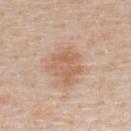Assessment: Captured during whole-body skin photography for melanoma surveillance; the lesion was not biopsied. Context: The tile uses white-light illumination. The patient is a male roughly 60 years of age. A 15 mm close-up tile from a total-body photography series done for melanoma screening. Measured at roughly 4.5 mm in maximum diameter. The lesion is located on the back.